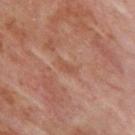The lesion was tiled from a total-body skin photograph and was not biopsied. The lesion-visualizer software estimated a border-irregularity index near 5/10, internal color variation of about 0 on a 0–10 scale, and radial color variation of about 0. The software also gave a nevus-likeness score of about 0/100 and a detector confidence of about 95 out of 100 that the crop contains a lesion. The lesion is located on the upper back. This is a cross-polarized tile. A 15 mm crop from a total-body photograph taken for skin-cancer surveillance. A male subject aged 68–72.